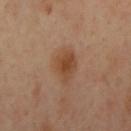  biopsy_status: not biopsied; imaged during a skin examination